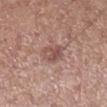This lesion was catalogued during total-body skin photography and was not selected for biopsy.
A 15 mm crop from a total-body photograph taken for skin-cancer surveillance.
A male subject, in their 60s.
The lesion is located on the right lower leg.